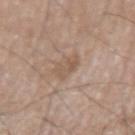Impression: This lesion was catalogued during total-body skin photography and was not selected for biopsy. Image and clinical context: A male patient, in their mid- to late 70s. A roughly 15 mm field-of-view crop from a total-body skin photograph. Located on the left upper arm.